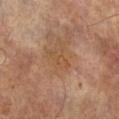The lesion was photographed on a routine skin check and not biopsied; there is no pathology result.
The tile uses cross-polarized illumination.
A close-up tile cropped from a whole-body skin photograph, about 15 mm across.
On the left lower leg.
A female subject aged 73 to 77.
Automated image analysis of the tile measured a lesion area of about 4 mm² and a shape eccentricity near 0.9. It also reported border irregularity of about 3.5 on a 0–10 scale and peripheral color asymmetry of about 0.5. The analysis additionally found a nevus-likeness score of about 0/100.
The recorded lesion diameter is about 3 mm.